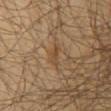{"biopsy_status": "not biopsied; imaged during a skin examination", "site": "mid back", "patient": {"sex": "male", "age_approx": 60}, "image": {"source": "total-body photography crop", "field_of_view_mm": 15}, "lesion_size": {"long_diameter_mm_approx": 2.5}, "lighting": "cross-polarized"}